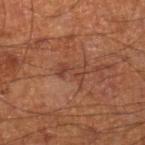follow-up: imaged on a skin check; not biopsied
image: ~15 mm crop, total-body skin-cancer survey
site: the leg
lesion diameter: about 3.5 mm
patient: male, aged 58–62
tile lighting: cross-polarized